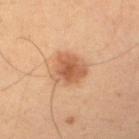Recorded during total-body skin imaging; not selected for excision or biopsy. A region of skin cropped from a whole-body photographic capture, roughly 15 mm wide. The subject is a male aged 48 to 52. Automated image analysis of the tile measured a normalized border contrast of about 7.5. The software also gave a border-irregularity index near 3/10. On the right upper arm. Measured at roughly 4 mm in maximum diameter. This is a cross-polarized tile.